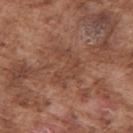Recorded during total-body skin imaging; not selected for excision or biopsy. The tile uses white-light illumination. The lesion's longest dimension is about 5 mm. A male patient, about 75 years old. A 15 mm crop from a total-body photograph taken for skin-cancer surveillance. The lesion is located on the right upper arm.